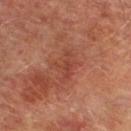Recorded during total-body skin imaging; not selected for excision or biopsy.
The patient is a male aged around 75.
From the leg.
The lesion-visualizer software estimated an area of roughly 7.5 mm², an outline eccentricity of about 0.75 (0 = round, 1 = elongated), and two-axis asymmetry of about 0.4. And it measured a classifier nevus-likeness of about 0/100 and a detector confidence of about 100 out of 100 that the crop contains a lesion.
Cropped from a total-body skin-imaging series; the visible field is about 15 mm.
The tile uses cross-polarized illumination.